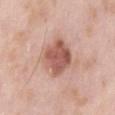notes: no biopsy performed (imaged during a skin exam) | tile lighting: white-light illumination | subject: male, aged 53–57 | body site: the front of the torso | image-analysis metrics: an eccentricity of roughly 0.45 and a shape-asymmetry score of about 0.15 (0 = symmetric); a border-irregularity rating of about 1.5/10, a within-lesion color-variation index near 4.5/10, and a peripheral color-asymmetry measure near 1.5; a classifier nevus-likeness of about 30/100 and lesion-presence confidence of about 100/100 | lesion size: ≈4 mm | acquisition: ~15 mm tile from a whole-body skin photo.